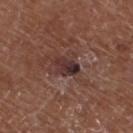Clinical impression: This lesion was catalogued during total-body skin photography and was not selected for biopsy. Background: The lesion is on the left lower leg. The lesion's longest dimension is about 3 mm. The subject is a male roughly 75 years of age. The lesion-visualizer software estimated border irregularity of about 2.5 on a 0–10 scale, a within-lesion color-variation index near 7/10, and a peripheral color-asymmetry measure near 2.5. And it measured an automated nevus-likeness rating near 0 out of 100 and a lesion-detection confidence of about 100/100. Cropped from a whole-body photographic skin survey; the tile spans about 15 mm.